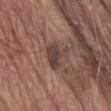{"lighting": "white-light", "patient": {"sex": "male", "age_approx": 80}, "site": "abdomen", "image": {"source": "total-body photography crop", "field_of_view_mm": 15}, "lesion_size": {"long_diameter_mm_approx": 3.5}, "automated_metrics": {"area_mm2_approx": 7.0, "shape_asymmetry": 0.4, "color_variation_0_10": 2.5, "peripheral_color_asymmetry": 1.0, "nevus_likeness_0_100": 0, "lesion_detection_confidence_0_100": 90}}